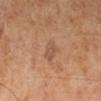No biopsy was performed on this lesion — it was imaged during a full skin examination and was not determined to be concerning.